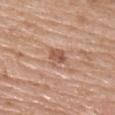biopsy status: total-body-photography surveillance lesion; no biopsy
image source: ~15 mm crop, total-body skin-cancer survey
automated lesion analysis: a mean CIELAB color near L≈56 a*≈22 b*≈30; a nevus-likeness score of about 5/100 and a lesion-detection confidence of about 100/100
lesion diameter: ≈2.5 mm
lighting: white-light
patient: female, about 65 years old
location: the upper back